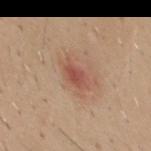follow-up = no biopsy performed (imaged during a skin exam) | patient = male, roughly 40 years of age | lighting = white-light illumination | anatomic site = the mid back | image source = 15 mm crop, total-body photography | lesion diameter = about 3 mm.